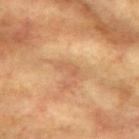Captured during whole-body skin photography for melanoma surveillance; the lesion was not biopsied. The tile uses cross-polarized illumination. Located on the left upper arm. A 15 mm close-up tile from a total-body photography series done for melanoma screening. The recorded lesion diameter is about 2.5 mm. A female patient in their mid-70s.